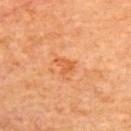This lesion was catalogued during total-body skin photography and was not selected for biopsy.
A 15 mm close-up extracted from a 3D total-body photography capture.
A female patient, in their mid-60s.
Measured at roughly 2.5 mm in maximum diameter.
Located on the back.
The total-body-photography lesion software estimated a nevus-likeness score of about 10/100 and a lesion-detection confidence of about 100/100.